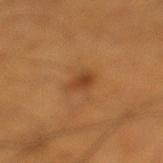Context: Captured under cross-polarized illumination. A male patient aged 53–57. A 15 mm crop from a total-body photograph taken for skin-cancer surveillance. About 2.5 mm across. On the left lower leg.